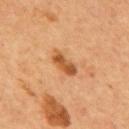Clinical impression: This lesion was catalogued during total-body skin photography and was not selected for biopsy. Image and clinical context: A male subject approximately 55 years of age. A close-up tile cropped from a whole-body skin photograph, about 15 mm across. From the mid back. The total-body-photography lesion software estimated a lesion–skin lightness drop of about 12. The tile uses cross-polarized illumination. About 3.5 mm across.